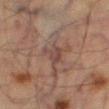This lesion was catalogued during total-body skin photography and was not selected for biopsy.